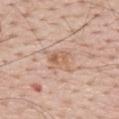workup: imaged on a skin check; not biopsied | anatomic site: the back | tile lighting: white-light | patient: male, aged around 65 | size: ≈3 mm | imaging modality: ~15 mm tile from a whole-body skin photo.